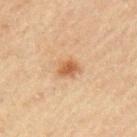Assessment: This lesion was catalogued during total-body skin photography and was not selected for biopsy. Clinical summary: The patient is a male about 45 years old. The lesion-visualizer software estimated a footprint of about 4 mm² and a shape eccentricity near 0.6. The software also gave a within-lesion color-variation index near 2.5/10 and a peripheral color-asymmetry measure near 1. The tile uses cross-polarized illumination. The lesion is located on the left upper arm. A region of skin cropped from a whole-body photographic capture, roughly 15 mm wide.